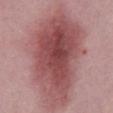notes: imaged on a skin check; not biopsied | subject: male, aged approximately 50 | lesion diameter: ~12.5 mm (longest diameter) | site: the abdomen | image: ~15 mm tile from a whole-body skin photo | automated metrics: an outline eccentricity of about 0.8 (0 = round, 1 = elongated) and two-axis asymmetry of about 0.25; a lesion color around L≈50 a*≈26 b*≈20 in CIELAB, about 13 CIELAB-L* units darker than the surrounding skin, and a normalized border contrast of about 9.5.